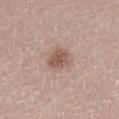The lesion was tiled from a total-body skin photograph and was not biopsied.
A roughly 15 mm field-of-view crop from a total-body skin photograph.
This is a white-light tile.
The patient is a female in their mid- to late 60s.
The lesion is on the front of the torso.
The lesion's longest dimension is about 3 mm.